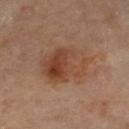Imaged during a routine full-body skin examination; the lesion was not biopsied and no histopathology is available. The subject is a female aged 58–62. A 15 mm close-up tile from a total-body photography series done for melanoma screening. Automated image analysis of the tile measured a footprint of about 21 mm² and a shape-asymmetry score of about 0.15 (0 = symmetric). The analysis additionally found a nevus-likeness score of about 90/100. From the leg.